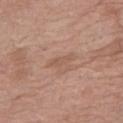Q: Was this lesion biopsied?
A: no biopsy performed (imaged during a skin exam)
Q: Patient demographics?
A: female, about 70 years old
Q: Illumination type?
A: white-light
Q: What kind of image is this?
A: total-body-photography crop, ~15 mm field of view
Q: Lesion location?
A: the right thigh
Q: How large is the lesion?
A: about 2.5 mm